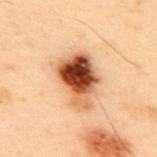{
  "biopsy_status": "not biopsied; imaged during a skin examination",
  "image": {
    "source": "total-body photography crop",
    "field_of_view_mm": 15
  },
  "site": "upper back",
  "patient": {
    "sex": "male",
    "age_approx": 55
  },
  "automated_metrics": {
    "area_mm2_approx": 16.0,
    "eccentricity": 0.75,
    "shape_asymmetry": 0.35,
    "nevus_likeness_0_100": 100,
    "lesion_detection_confidence_0_100": 100
  }
}